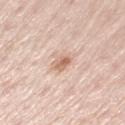lesion diameter=≈2.5 mm
image-analysis metrics=a border-irregularity rating of about 2/10 and radial color variation of about 1.5; a nevus-likeness score of about 35/100 and a detector confidence of about 100 out of 100 that the crop contains a lesion
image=~15 mm crop, total-body skin-cancer survey
patient=female, aged approximately 50
location=the left upper arm